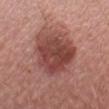- notes — catalogued during a skin exam; not biopsied
- size — ~6 mm (longest diameter)
- subject — male, roughly 30 years of age
- image — ~15 mm tile from a whole-body skin photo
- illumination — white-light